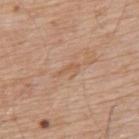Assessment:
No biopsy was performed on this lesion — it was imaged during a full skin examination and was not determined to be concerning.